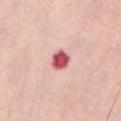Case summary:
– workup · imaged on a skin check; not biopsied
– site · the abdomen
– image source · total-body-photography crop, ~15 mm field of view
– patient · female, in their 70s